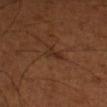biopsy_status: not biopsied; imaged during a skin examination
lesion_size:
  long_diameter_mm_approx: 2.5
automated_metrics:
  eccentricity: 0.8
  border_irregularity_0_10: 5.5
  color_variation_0_10: 0.0
  peripheral_color_asymmetry: 0.0
image:
  source: total-body photography crop
  field_of_view_mm: 15
site: left upper arm
patient:
  sex: male
  age_approx: 50
lighting: cross-polarized